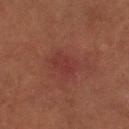Impression:
Imaged during a routine full-body skin examination; the lesion was not biopsied and no histopathology is available.
Background:
A 15 mm crop from a total-body photograph taken for skin-cancer surveillance. The patient is a female aged 78 to 82. Located on the leg. Imaged with cross-polarized lighting. The recorded lesion diameter is about 3.5 mm.